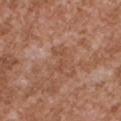Impression: This lesion was catalogued during total-body skin photography and was not selected for biopsy. Acquisition and patient details: The lesion is on the chest. A 15 mm crop from a total-body photograph taken for skin-cancer surveillance. The subject is a male roughly 45 years of age. Approximately 3.5 mm at its widest. Captured under white-light illumination.